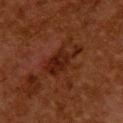Clinical impression: Recorded during total-body skin imaging; not selected for excision or biopsy. Clinical summary: A roughly 15 mm field-of-view crop from a total-body skin photograph. The lesion is located on the upper back. Longest diameter approximately 5.5 mm. Automated tile analysis of the lesion measured a footprint of about 10 mm², an eccentricity of roughly 0.9, and a shape-asymmetry score of about 0.45 (0 = symmetric). The analysis additionally found a within-lesion color-variation index near 3.5/10 and radial color variation of about 1. And it measured an automated nevus-likeness rating near 75 out of 100 and a detector confidence of about 100 out of 100 that the crop contains a lesion. The patient is a female aged around 50. Imaged with cross-polarized lighting.